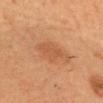This lesion was catalogued during total-body skin photography and was not selected for biopsy. From the head or neck. Imaged with cross-polarized lighting. The total-body-photography lesion software estimated a lesion area of about 6 mm², an eccentricity of roughly 0.7, and two-axis asymmetry of about 0.2. The analysis additionally found a within-lesion color-variation index near 1.5/10 and a peripheral color-asymmetry measure near 0.5. The software also gave a classifier nevus-likeness of about 45/100 and a lesion-detection confidence of about 100/100. A 15 mm close-up tile from a total-body photography series done for melanoma screening. A male patient, approximately 40 years of age.